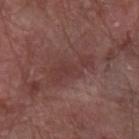A male subject, approximately 80 years of age. Automated tile analysis of the lesion measured a lesion color around L≈37 a*≈22 b*≈20 in CIELAB, about 6 CIELAB-L* units darker than the surrounding skin, and a normalized lesion–skin contrast near 5.5. And it measured a within-lesion color-variation index near 2/10 and radial color variation of about 0.5. And it measured an automated nevus-likeness rating near 0 out of 100. This is a white-light tile. The lesion is on the arm. A close-up tile cropped from a whole-body skin photograph, about 15 mm across.